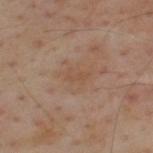The lesion was photographed on a routine skin check and not biopsied; there is no pathology result. A close-up tile cropped from a whole-body skin photograph, about 15 mm across. The subject is a male aged 53 to 57. The recorded lesion diameter is about 2.5 mm. Captured under cross-polarized illumination. The lesion is located on the upper back. The lesion-visualizer software estimated a footprint of about 3 mm², a shape eccentricity near 0.85, and a shape-asymmetry score of about 0.35 (0 = symmetric). It also reported a lesion color around L≈49 a*≈18 b*≈29 in CIELAB. And it measured border irregularity of about 4 on a 0–10 scale, internal color variation of about 0 on a 0–10 scale, and a peripheral color-asymmetry measure near 0. The software also gave an automated nevus-likeness rating near 0 out of 100.